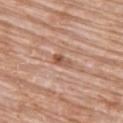Impression: The lesion was photographed on a routine skin check and not biopsied; there is no pathology result. Clinical summary: Located on the upper back. A female subject aged 83 or older. A 15 mm crop from a total-body photograph taken for skin-cancer surveillance. The tile uses white-light illumination. Automated image analysis of the tile measured radial color variation of about 0. The software also gave a classifier nevus-likeness of about 0/100 and a detector confidence of about 100 out of 100 that the crop contains a lesion. The lesion's longest dimension is about 2.5 mm.